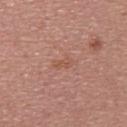Background:
About 2.5 mm across. A female subject in their mid- to late 30s. A lesion tile, about 15 mm wide, cut from a 3D total-body photograph. On the back.